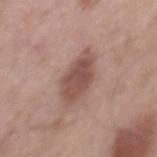Q: Was this lesion biopsied?
A: imaged on a skin check; not biopsied
Q: How was the tile lit?
A: white-light
Q: What did automated image analysis measure?
A: a lesion area of about 13 mm², an outline eccentricity of about 0.85 (0 = round, 1 = elongated), and a symmetry-axis asymmetry near 0.15; a lesion color around L≈51 a*≈20 b*≈24 in CIELAB and roughly 11 lightness units darker than nearby skin
Q: Where on the body is the lesion?
A: the mid back
Q: What is the imaging modality?
A: total-body-photography crop, ~15 mm field of view
Q: What are the patient's age and sex?
A: male, in their mid- to late 50s
Q: What is the lesion's diameter?
A: ≈6 mm